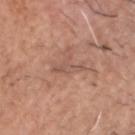Clinical impression: The lesion was photographed on a routine skin check and not biopsied; there is no pathology result. Image and clinical context: A 15 mm crop from a total-body photograph taken for skin-cancer surveillance. The lesion is on the head or neck. A male subject aged 68 to 72.